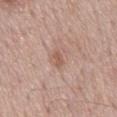notes = no biopsy performed (imaged during a skin exam) | image = ~15 mm tile from a whole-body skin photo | body site = the mid back | patient = male, about 70 years old.